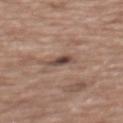workup=imaged on a skin check; not biopsied | subject=female, about 75 years old | image=~15 mm tile from a whole-body skin photo | location=the back.